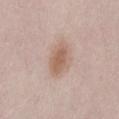Assessment: Imaged during a routine full-body skin examination; the lesion was not biopsied and no histopathology is available. Background: Automated image analysis of the tile measured a lesion-detection confidence of about 100/100. A close-up tile cropped from a whole-body skin photograph, about 15 mm across. Approximately 4 mm at its widest. A female patient aged around 50. On the lower back. Captured under white-light illumination.